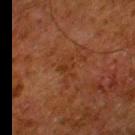No biopsy was performed on this lesion — it was imaged during a full skin examination and was not determined to be concerning.
Automated image analysis of the tile measured a mean CIELAB color near L≈26 a*≈19 b*≈28, a lesion–skin lightness drop of about 4, and a normalized lesion–skin contrast near 5. The software also gave border irregularity of about 4.5 on a 0–10 scale and peripheral color asymmetry of about 0.5.
A 15 mm close-up tile from a total-body photography series done for melanoma screening.
Captured under cross-polarized illumination.
The recorded lesion diameter is about 3 mm.
The lesion is located on the left thigh.
A male subject, roughly 80 years of age.